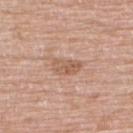The lesion was photographed on a routine skin check and not biopsied; there is no pathology result.